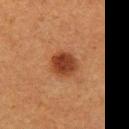{
  "image": {
    "source": "total-body photography crop",
    "field_of_view_mm": 15
  },
  "site": "left thigh",
  "patient": {
    "sex": "female",
    "age_approx": 40
  }
}